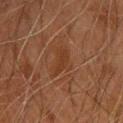Clinical impression:
This lesion was catalogued during total-body skin photography and was not selected for biopsy.
Background:
Longest diameter approximately 3 mm. Automated image analysis of the tile measured an average lesion color of about L≈30 a*≈20 b*≈28 (CIELAB), a lesion–skin lightness drop of about 5, and a lesion-to-skin contrast of about 5.5 (normalized; higher = more distinct). The analysis additionally found a classifier nevus-likeness of about 5/100 and a lesion-detection confidence of about 100/100. A male subject roughly 45 years of age. The lesion is on the chest. A region of skin cropped from a whole-body photographic capture, roughly 15 mm wide.